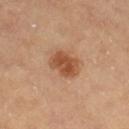Acquisition and patient details: A female subject, roughly 60 years of age. The lesion is on the right lower leg. This image is a 15 mm lesion crop taken from a total-body photograph.